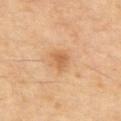workup: catalogued during a skin exam; not biopsied | location: the upper back | subject: male, about 55 years old | lesion diameter: ~2.5 mm (longest diameter) | image: ~15 mm tile from a whole-body skin photo.